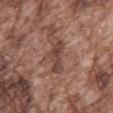<tbp_lesion>
  <biopsy_status>not biopsied; imaged during a skin examination</biopsy_status>
  <site>mid back</site>
  <image>
    <source>total-body photography crop</source>
    <field_of_view_mm>15</field_of_view_mm>
  </image>
  <automated_metrics>
    <cielab_L>43</cielab_L>
    <cielab_a>19</cielab_a>
    <cielab_b>24</cielab_b>
    <vs_skin_darker_L>10.0</vs_skin_darker_L>
    <vs_skin_contrast_norm>8.0</vs_skin_contrast_norm>
  </automated_metrics>
  <patient>
    <sex>male</sex>
    <age_approx>75</age_approx>
  </patient>
  <lighting>white-light</lighting>
  <lesion_size>
    <long_diameter_mm_approx>4.5</long_diameter_mm_approx>
  </lesion_size>
</tbp_lesion>